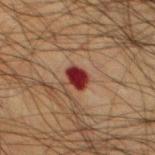Part of a total-body skin-imaging series; this lesion was reviewed on a skin check and was not flagged for biopsy.
On the front of the torso.
Cropped from a total-body skin-imaging series; the visible field is about 15 mm.
Measured at roughly 2.5 mm in maximum diameter.
Captured under cross-polarized illumination.
The subject is a male approximately 65 years of age.